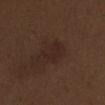follow-up = catalogued during a skin exam; not biopsied
subject = male, roughly 70 years of age
site = the abdomen
imaging modality = total-body-photography crop, ~15 mm field of view
lesion size = about 3.5 mm
illumination = white-light illumination
TBP lesion metrics = a classifier nevus-likeness of about 0/100 and a lesion-detection confidence of about 100/100